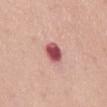Captured during whole-body skin photography for melanoma surveillance; the lesion was not biopsied. On the mid back. A region of skin cropped from a whole-body photographic capture, roughly 15 mm wide. A female patient, in their mid-60s. Longest diameter approximately 3 mm.